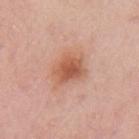Assessment:
This lesion was catalogued during total-body skin photography and was not selected for biopsy.
Background:
From the left upper arm. A female patient, roughly 40 years of age. Imaged with white-light lighting. A 15 mm close-up tile from a total-body photography series done for melanoma screening. Measured at roughly 4 mm in maximum diameter.